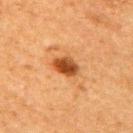Clinical impression:
The lesion was tiled from a total-body skin photograph and was not biopsied.
Clinical summary:
The lesion is on the right upper arm. Captured under cross-polarized illumination. The lesion's longest dimension is about 3 mm. Cropped from a total-body skin-imaging series; the visible field is about 15 mm. Automated image analysis of the tile measured about 14 CIELAB-L* units darker than the surrounding skin and a lesion-to-skin contrast of about 10.5 (normalized; higher = more distinct). And it measured border irregularity of about 1.5 on a 0–10 scale, a within-lesion color-variation index near 4.5/10, and a peripheral color-asymmetry measure near 1.5. A female subject, about 60 years old.